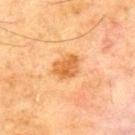Findings:
• biopsy status — catalogued during a skin exam; not biopsied
• body site — the upper back
• image source — total-body-photography crop, ~15 mm field of view
• illumination — cross-polarized
• lesion size — ~3.5 mm (longest diameter)
• patient — male, aged around 70